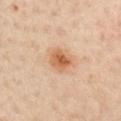Assessment:
This lesion was catalogued during total-body skin photography and was not selected for biopsy.
Context:
Imaged with cross-polarized lighting. The total-body-photography lesion software estimated an eccentricity of roughly 0.5 and a shape-asymmetry score of about 0.2 (0 = symmetric). It also reported a mean CIELAB color near L≈62 a*≈23 b*≈37 and a lesion-to-skin contrast of about 8.5 (normalized; higher = more distinct). And it measured a nevus-likeness score of about 95/100. Longest diameter approximately 3 mm. The patient is a male aged 63–67. From the chest. A region of skin cropped from a whole-body photographic capture, roughly 15 mm wide.